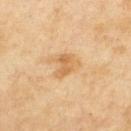Case summary:
• follow-up: no biopsy performed (imaged during a skin exam)
• acquisition: total-body-photography crop, ~15 mm field of view
• location: the upper back
• patient: female, aged approximately 60
• size: ~3 mm (longest diameter)